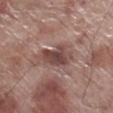Notes:
- biopsy status — no biopsy performed (imaged during a skin exam)
- patient — male, aged 68–72
- body site — the right lower leg
- acquisition — ~15 mm tile from a whole-body skin photo